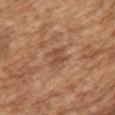Recorded during total-body skin imaging; not selected for excision or biopsy. Located on the upper back. Cropped from a whole-body photographic skin survey; the tile spans about 15 mm. The lesion's longest dimension is about 3 mm. This is a white-light tile. The lesion-visualizer software estimated a lesion area of about 5 mm² and two-axis asymmetry of about 0.25. The analysis additionally found an average lesion color of about L≈49 a*≈22 b*≈31 (CIELAB), a lesion–skin lightness drop of about 8, and a normalized border contrast of about 6.5. It also reported a border-irregularity rating of about 3.5/10 and internal color variation of about 2.5 on a 0–10 scale. The patient is a female aged around 30.